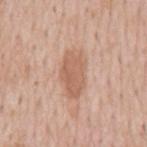follow-up — catalogued during a skin exam; not biopsied
TBP lesion metrics — a lesion area of about 12 mm², a shape eccentricity near 0.85, and a shape-asymmetry score of about 0.15 (0 = symmetric); a mean CIELAB color near L≈61 a*≈21 b*≈31, about 9 CIELAB-L* units darker than the surrounding skin, and a lesion-to-skin contrast of about 6.5 (normalized; higher = more distinct); a border-irregularity index near 2/10, a within-lesion color-variation index near 2.5/10, and radial color variation of about 1
illumination — white-light illumination
anatomic site — the chest
patient — male, aged 58 to 62
image — ~15 mm crop, total-body skin-cancer survey
lesion size — ~5.5 mm (longest diameter)